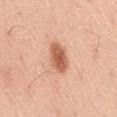workup=total-body-photography surveillance lesion; no biopsy
imaging modality=total-body-photography crop, ~15 mm field of view
subject=male, roughly 50 years of age
anatomic site=the right thigh